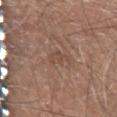Assessment:
This lesion was catalogued during total-body skin photography and was not selected for biopsy.
Image and clinical context:
On the left forearm. Automated image analysis of the tile measured a border-irregularity index near 5/10 and a peripheral color-asymmetry measure near 0.5. It also reported a classifier nevus-likeness of about 0/100. A 15 mm close-up extracted from a 3D total-body photography capture. The subject is a male aged around 70. The tile uses white-light illumination. About 2.5 mm across.